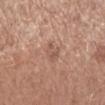The lesion was tiled from a total-body skin photograph and was not biopsied. A female patient aged around 55. The lesion is located on the left forearm. Captured under white-light illumination. An algorithmic analysis of the crop reported a footprint of about 3.5 mm², a shape eccentricity near 0.8, and a symmetry-axis asymmetry near 0.3. The analysis additionally found a nevus-likeness score of about 0/100 and a lesion-detection confidence of about 100/100. The lesion's longest dimension is about 2.5 mm. Cropped from a total-body skin-imaging series; the visible field is about 15 mm.